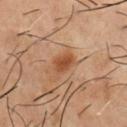workup — total-body-photography surveillance lesion; no biopsy
illumination — cross-polarized illumination
lesion diameter — ≈2.5 mm
subject — male, aged approximately 55
automated lesion analysis — a mean CIELAB color near L≈48 a*≈24 b*≈35, about 10 CIELAB-L* units darker than the surrounding skin, and a normalized border contrast of about 8.5; a classifier nevus-likeness of about 90/100
imaging modality — ~15 mm tile from a whole-body skin photo
body site — the chest